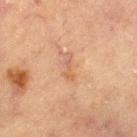follow-up: no biopsy performed (imaged during a skin exam) | diameter: about 3 mm | subject: male, aged around 65 | body site: the leg | image source: ~15 mm crop, total-body skin-cancer survey | TBP lesion metrics: an area of roughly 2.5 mm², an outline eccentricity of about 0.95 (0 = round, 1 = elongated), and a symmetry-axis asymmetry near 0.5; a mean CIELAB color near L≈48 a*≈18 b*≈28, about 6 CIELAB-L* units darker than the surrounding skin, and a normalized lesion–skin contrast near 5; a border-irregularity rating of about 6.5/10, internal color variation of about 0 on a 0–10 scale, and a peripheral color-asymmetry measure near 0; a nevus-likeness score of about 0/100 and a lesion-detection confidence of about 100/100.